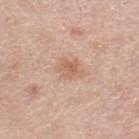biopsy status: imaged on a skin check; not biopsied | patient: female, aged approximately 65 | image-analysis metrics: an average lesion color of about L≈62 a*≈19 b*≈31 (CIELAB), a lesion–skin lightness drop of about 8, and a normalized border contrast of about 6; border irregularity of about 3 on a 0–10 scale, a within-lesion color-variation index near 3.5/10, and radial color variation of about 1 | acquisition: total-body-photography crop, ~15 mm field of view | body site: the right lower leg | tile lighting: white-light.